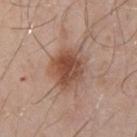workup = total-body-photography surveillance lesion; no biopsy
patient = male, aged 28 to 32
image source = total-body-photography crop, ~15 mm field of view
location = the back